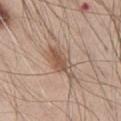Imaged during a routine full-body skin examination; the lesion was not biopsied and no histopathology is available.
Imaged with white-light lighting.
About 5 mm across.
A 15 mm close-up extracted from a 3D total-body photography capture.
On the chest.
The patient is a male roughly 45 years of age.